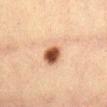Clinical summary:
The tile uses cross-polarized illumination. A female patient, about 40 years old. A region of skin cropped from a whole-body photographic capture, roughly 15 mm wide. From the front of the torso.